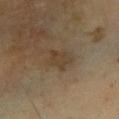The lesion was tiled from a total-body skin photograph and was not biopsied. A close-up tile cropped from a whole-body skin photograph, about 15 mm across. Longest diameter approximately 3.5 mm. On the left lower leg. A male patient, approximately 65 years of age. Imaged with cross-polarized lighting.